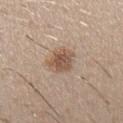Part of a total-body skin-imaging series; this lesion was reviewed on a skin check and was not flagged for biopsy.
Automated image analysis of the tile measured an average lesion color of about L≈54 a*≈17 b*≈28 (CIELAB) and about 11 CIELAB-L* units darker than the surrounding skin. And it measured a border-irregularity rating of about 2.5/10, internal color variation of about 3 on a 0–10 scale, and radial color variation of about 1. The analysis additionally found a nevus-likeness score of about 90/100 and a lesion-detection confidence of about 100/100.
The lesion is on the arm.
A male patient, in their 30s.
This is a white-light tile.
A 15 mm crop from a total-body photograph taken for skin-cancer surveillance.